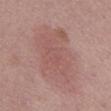| key | value |
|---|---|
| workup | no biopsy performed (imaged during a skin exam) |
| illumination | white-light |
| anatomic site | the mid back |
| subject | female, in their mid- to late 60s |
| image | ~15 mm crop, total-body skin-cancer survey |
| size | ≈9.5 mm |